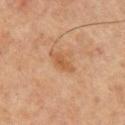| key | value |
|---|---|
| notes | total-body-photography surveillance lesion; no biopsy |
| lesion size | about 3 mm |
| patient | male, aged approximately 65 |
| image | ~15 mm crop, total-body skin-cancer survey |
| body site | the back |
| illumination | cross-polarized illumination |